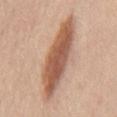notes: imaged on a skin check; not biopsied
site: the mid back
image source: total-body-photography crop, ~15 mm field of view
patient: male, aged around 60
illumination: white-light
automated metrics: an area of roughly 26 mm², an eccentricity of roughly 0.95, and a shape-asymmetry score of about 0.2 (0 = symmetric); a classifier nevus-likeness of about 90/100 and lesion-presence confidence of about 100/100
lesion size: ~10 mm (longest diameter)